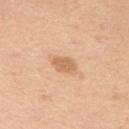| feature | finding |
|---|---|
| notes | no biopsy performed (imaged during a skin exam) |
| image source | ~15 mm tile from a whole-body skin photo |
| location | the right upper arm |
| illumination | white-light |
| patient | female, aged approximately 40 |
| diameter | ~2.5 mm (longest diameter) |